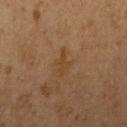Findings:
* follow-up · no biopsy performed (imaged during a skin exam)
* patient · female, aged 18–22
* imaging modality · ~15 mm crop, total-body skin-cancer survey
* body site · the arm
* lesion size · ≈2.5 mm
* illumination · cross-polarized
* image-analysis metrics · a lesion area of about 3 mm² and a shape eccentricity near 0.85; a border-irregularity rating of about 5.5/10, a color-variation rating of about 0/10, and radial color variation of about 0; a nevus-likeness score of about 0/100 and a detector confidence of about 100 out of 100 that the crop contains a lesion